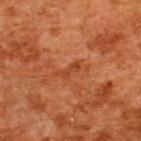No biopsy was performed on this lesion — it was imaged during a full skin examination and was not determined to be concerning. A male patient about 60 years old. The tile uses cross-polarized illumination. This image is a 15 mm lesion crop taken from a total-body photograph. Automated tile analysis of the lesion measured an area of roughly 2.5 mm² and a shape-asymmetry score of about 0.4 (0 = symmetric). It also reported an automated nevus-likeness rating near 0 out of 100 and a lesion-detection confidence of about 100/100. Measured at roughly 3 mm in maximum diameter. The lesion is on the upper back.